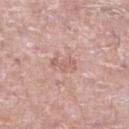Background: Captured under white-light illumination. About 3 mm across. The total-body-photography lesion software estimated a lesion area of about 5 mm² and two-axis asymmetry of about 0.3. A male patient, in their 50s. Located on the right lower leg. A 15 mm crop from a total-body photograph taken for skin-cancer surveillance.